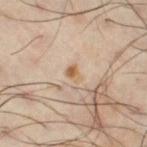Background: About 2 mm across. This is a cross-polarized tile. A 15 mm close-up extracted from a 3D total-body photography capture. The patient is a male aged 38 to 42. The lesion is located on the right thigh. An algorithmic analysis of the crop reported border irregularity of about 2.5 on a 0–10 scale, a within-lesion color-variation index near 3/10, and peripheral color asymmetry of about 1.